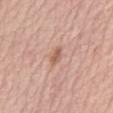Recorded during total-body skin imaging; not selected for excision or biopsy. A roughly 15 mm field-of-view crop from a total-body skin photograph. The lesion is located on the mid back. A female patient aged 63 to 67. The tile uses white-light illumination. Measured at roughly 2.5 mm in maximum diameter.